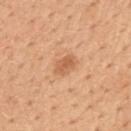Notes:
* workup · total-body-photography surveillance lesion; no biopsy
* body site · the back
* tile lighting · white-light
* subject · male, roughly 50 years of age
* size · ~3 mm (longest diameter)
* acquisition · ~15 mm crop, total-body skin-cancer survey